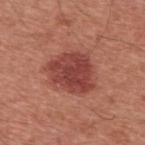Clinical summary: The patient is a male aged approximately 60. A region of skin cropped from a whole-body photographic capture, roughly 15 mm wide. Located on the back.